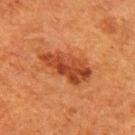Q: Was this lesion biopsied?
A: catalogued during a skin exam; not biopsied
Q: Lesion size?
A: ~6 mm (longest diameter)
Q: What did automated image analysis measure?
A: a mean CIELAB color near L≈38 a*≈27 b*≈34, a lesion–skin lightness drop of about 10, and a lesion-to-skin contrast of about 8.5 (normalized; higher = more distinct); internal color variation of about 5.5 on a 0–10 scale and peripheral color asymmetry of about 2; a nevus-likeness score of about 55/100 and lesion-presence confidence of about 100/100
Q: What is the anatomic site?
A: the right upper arm
Q: Who is the patient?
A: female, aged 48–52
Q: What kind of image is this?
A: 15 mm crop, total-body photography
Q: How was the tile lit?
A: cross-polarized illumination